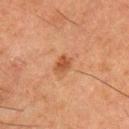Q: Was a biopsy performed?
A: no biopsy performed (imaged during a skin exam)
Q: What kind of image is this?
A: ~15 mm crop, total-body skin-cancer survey
Q: Who is the patient?
A: male, about 50 years old
Q: What is the anatomic site?
A: the left upper arm